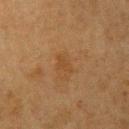No biopsy was performed on this lesion — it was imaged during a full skin examination and was not determined to be concerning. A female subject, roughly 55 years of age. Imaged with cross-polarized lighting. Located on the left upper arm. A 15 mm close-up tile from a total-body photography series done for melanoma screening.